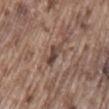No biopsy was performed on this lesion — it was imaged during a full skin examination and was not determined to be concerning.
Captured under white-light illumination.
The lesion is on the back.
A 15 mm close-up tile from a total-body photography series done for melanoma screening.
The patient is a male in their mid-70s.
Approximately 3 mm at its widest.